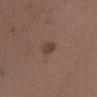  biopsy_status: not biopsied; imaged during a skin examination
  site: head or neck
  lesion_size:
    long_diameter_mm_approx: 2.5
  automated_metrics:
    area_mm2_approx: 3.5
    eccentricity: 0.75
    cielab_L: 41
    cielab_a: 18
    cielab_b: 26
    vs_skin_darker_L: 8.0
    vs_skin_contrast_norm: 7.0
    color_variation_0_10: 2.0
    peripheral_color_asymmetry: 0.5
    nevus_likeness_0_100: 75
    lesion_detection_confidence_0_100: 100
  lighting: white-light
  image:
    source: total-body photography crop
    field_of_view_mm: 15
  patient:
    sex: female
    age_approx: 30